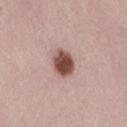This lesion was catalogued during total-body skin photography and was not selected for biopsy.
An algorithmic analysis of the crop reported a lesion area of about 7 mm², a shape eccentricity near 0.5, and a symmetry-axis asymmetry near 0.1.
A 15 mm close-up tile from a total-body photography series done for melanoma screening.
From the right thigh.
The tile uses white-light illumination.
A male subject, approximately 45 years of age.